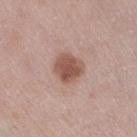Imaged during a routine full-body skin examination; the lesion was not biopsied and no histopathology is available. Measured at roughly 3.5 mm in maximum diameter. On the right thigh. The tile uses white-light illumination. A 15 mm crop from a total-body photograph taken for skin-cancer surveillance. A female patient aged around 40.